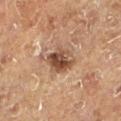This lesion was catalogued during total-body skin photography and was not selected for biopsy.
The subject is a male approximately 75 years of age.
About 4 mm across.
The total-body-photography lesion software estimated a footprint of about 8.5 mm², an eccentricity of roughly 0.6, and a symmetry-axis asymmetry near 0.2. It also reported a border-irregularity rating of about 2/10, a color-variation rating of about 7/10, and a peripheral color-asymmetry measure near 2.5.
A 15 mm close-up tile from a total-body photography series done for melanoma screening.
The lesion is located on the leg.
Imaged with cross-polarized lighting.